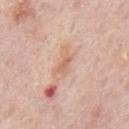patient:
  sex: male
  age_approx: 75
image:
  source: total-body photography crop
  field_of_view_mm: 15
site: mid back